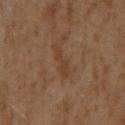This lesion was catalogued during total-body skin photography and was not selected for biopsy.
A roughly 15 mm field-of-view crop from a total-body skin photograph.
Measured at roughly 4 mm in maximum diameter.
The lesion is located on the back.
A male patient approximately 60 years of age.